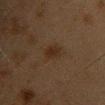Case summary:
- size · ≈2.5 mm
- illumination · cross-polarized
- site · the front of the torso
- patient · female, in their 40s
- acquisition · ~15 mm crop, total-body skin-cancer survey